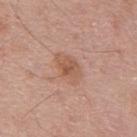An algorithmic analysis of the crop reported a lesion area of about 8.5 mm² and a shape-asymmetry score of about 0.15 (0 = symmetric). The analysis additionally found a nevus-likeness score of about 15/100 and a lesion-detection confidence of about 100/100.
A male subject roughly 45 years of age.
Located on the upper back.
Approximately 4 mm at its widest.
A 15 mm close-up extracted from a 3D total-body photography capture.